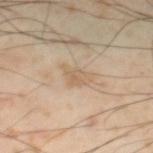Recorded during total-body skin imaging; not selected for excision or biopsy. This image is a 15 mm lesion crop taken from a total-body photograph. A male patient aged approximately 55. On the leg.